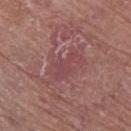Findings:
* biopsy status: catalogued during a skin exam; not biopsied
* acquisition: ~15 mm crop, total-body skin-cancer survey
* location: the leg
* patient: female, aged approximately 80
* size: about 4 mm
* lighting: white-light
* TBP lesion metrics: a footprint of about 6 mm², a shape eccentricity near 0.85, and a shape-asymmetry score of about 0.4 (0 = symmetric); a mean CIELAB color near L≈45 a*≈25 b*≈19 and roughly 6 lightness units darker than nearby skin